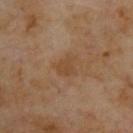Q: Was this lesion biopsied?
A: imaged on a skin check; not biopsied
Q: Illumination type?
A: cross-polarized
Q: What kind of image is this?
A: total-body-photography crop, ~15 mm field of view
Q: What did automated image analysis measure?
A: an area of roughly 5 mm², an eccentricity of roughly 0.6, and a symmetry-axis asymmetry near 0.35; roughly 6 lightness units darker than nearby skin and a lesion-to-skin contrast of about 5.5 (normalized; higher = more distinct); a border-irregularity rating of about 3.5/10, a color-variation rating of about 2/10, and a peripheral color-asymmetry measure near 1; an automated nevus-likeness rating near 0 out of 100
Q: Who is the patient?
A: male, approximately 70 years of age
Q: What is the anatomic site?
A: the upper back
Q: What is the lesion's diameter?
A: about 3 mm